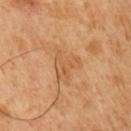Assessment: The lesion was photographed on a routine skin check and not biopsied; there is no pathology result. Acquisition and patient details: Longest diameter approximately 5 mm. Located on the right upper arm. A 15 mm close-up extracted from a 3D total-body photography capture. A male patient, aged 48 to 52. The total-body-photography lesion software estimated a mean CIELAB color near L≈57 a*≈21 b*≈39, a lesion–skin lightness drop of about 7, and a lesion-to-skin contrast of about 5 (normalized; higher = more distinct). The software also gave a border-irregularity rating of about 6.5/10, a within-lesion color-variation index near 3/10, and a peripheral color-asymmetry measure near 1. Imaged with cross-polarized lighting.